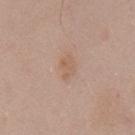Clinical impression:
Part of a total-body skin-imaging series; this lesion was reviewed on a skin check and was not flagged for biopsy.
Acquisition and patient details:
A male patient approximately 55 years of age. This is a white-light tile. About 3 mm across. A 15 mm close-up tile from a total-body photography series done for melanoma screening. Located on the chest.